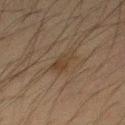Q: Was this lesion biopsied?
A: catalogued during a skin exam; not biopsied
Q: Illumination type?
A: cross-polarized illumination
Q: How was this image acquired?
A: total-body-photography crop, ~15 mm field of view
Q: Lesion location?
A: the front of the torso
Q: Patient demographics?
A: male, about 35 years old